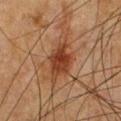Impression: This lesion was catalogued during total-body skin photography and was not selected for biopsy. Background: A male patient, aged 48–52. About 3.5 mm across. The total-body-photography lesion software estimated a classifier nevus-likeness of about 90/100 and a lesion-detection confidence of about 100/100. This image is a 15 mm lesion crop taken from a total-body photograph. The lesion is on the front of the torso.